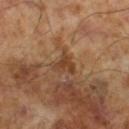Assessment: The lesion was tiled from a total-body skin photograph and was not biopsied. Clinical summary: Imaged with cross-polarized lighting. A male patient, about 65 years old. The lesion-visualizer software estimated roughly 9 lightness units darker than nearby skin and a lesion-to-skin contrast of about 7 (normalized; higher = more distinct). A region of skin cropped from a whole-body photographic capture, roughly 15 mm wide.